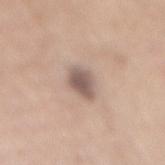Clinical impression: Imaged during a routine full-body skin examination; the lesion was not biopsied and no histopathology is available. Context: On the back. A close-up tile cropped from a whole-body skin photograph, about 15 mm across. The recorded lesion diameter is about 3.5 mm. The total-body-photography lesion software estimated a lesion area of about 6 mm², an outline eccentricity of about 0.75 (0 = round, 1 = elongated), and a symmetry-axis asymmetry near 0.15. And it measured a lesion color around L≈56 a*≈15 b*≈22 in CIELAB, about 13 CIELAB-L* units darker than the surrounding skin, and a normalized border contrast of about 9. This is a white-light tile. A female subject roughly 60 years of age.